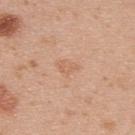biopsy_status: not biopsied; imaged during a skin examination
lesion_size:
  long_diameter_mm_approx: 2.5
image:
  source: total-body photography crop
  field_of_view_mm: 15
automated_metrics:
  cielab_L: 63
  cielab_a: 20
  cielab_b: 34
  vs_skin_contrast_norm: 4.5
lighting: white-light
site: upper back
patient:
  sex: male
  age_approx: 35